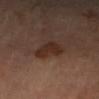Notes:
* TBP lesion metrics — an area of roughly 5.5 mm², a shape eccentricity near 0.8, and a shape-asymmetry score of about 0.45 (0 = symmetric); an average lesion color of about L≈28 a*≈18 b*≈24 (CIELAB), roughly 8 lightness units darker than nearby skin, and a normalized border contrast of about 8.5; a lesion-detection confidence of about 100/100
* subject — female, in their mid-60s
* tile lighting — cross-polarized illumination
* anatomic site — the left forearm
* size — ~3.5 mm (longest diameter)
* imaging modality — ~15 mm crop, total-body skin-cancer survey